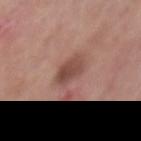notes: imaged on a skin check; not biopsied
subject: female, roughly 65 years of age
acquisition: total-body-photography crop, ~15 mm field of view
automated lesion analysis: a footprint of about 8 mm², an outline eccentricity of about 0.55 (0 = round, 1 = elongated), and a shape-asymmetry score of about 0.15 (0 = symmetric)
body site: the chest
size: about 3.5 mm
tile lighting: white-light